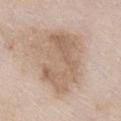The lesion was photographed on a routine skin check and not biopsied; there is no pathology result.
A female subject, in their mid-70s.
A 15 mm close-up extracted from a 3D total-body photography capture.
Longest diameter approximately 8 mm.
The lesion is on the chest.
The lesion-visualizer software estimated a lesion color around L≈62 a*≈15 b*≈28 in CIELAB and a lesion-to-skin contrast of about 6 (normalized; higher = more distinct). The software also gave a classifier nevus-likeness of about 15/100.